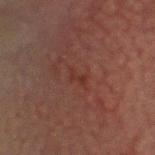Imaged during a routine full-body skin examination; the lesion was not biopsied and no histopathology is available. Measured at roughly 2.5 mm in maximum diameter. Automated image analysis of the tile measured an average lesion color of about L≈27 a*≈20 b*≈21 (CIELAB), about 4 CIELAB-L* units darker than the surrounding skin, and a lesion-to-skin contrast of about 5 (normalized; higher = more distinct). It also reported a classifier nevus-likeness of about 0/100 and a detector confidence of about 100 out of 100 that the crop contains a lesion. Located on the head or neck. A 15 mm close-up tile from a total-body photography series done for melanoma screening. The tile uses cross-polarized illumination. The patient is a male approximately 70 years of age.